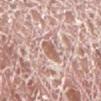Imaged during a routine full-body skin examination; the lesion was not biopsied and no histopathology is available.
Captured under white-light illumination.
The lesion is located on the right lower leg.
Cropped from a total-body skin-imaging series; the visible field is about 15 mm.
About 2.5 mm across.
A male patient, aged around 75.